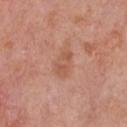The lesion was tiled from a total-body skin photograph and was not biopsied. A male patient, approximately 80 years of age. A lesion tile, about 15 mm wide, cut from a 3D total-body photograph. The lesion is located on the front of the torso.